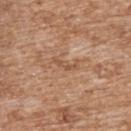{
  "biopsy_status": "not biopsied; imaged during a skin examination",
  "image": {
    "source": "total-body photography crop",
    "field_of_view_mm": 15
  },
  "lesion_size": {
    "long_diameter_mm_approx": 2.5
  },
  "lighting": "white-light",
  "patient": {
    "sex": "female",
    "age_approx": 70
  },
  "site": "upper back",
  "automated_metrics": {
    "eccentricity": 0.95,
    "shape_asymmetry": 0.55,
    "cielab_L": 53,
    "cielab_a": 20,
    "cielab_b": 33,
    "vs_skin_darker_L": 8.0,
    "vs_skin_contrast_norm": 5.5,
    "border_irregularity_0_10": 6.0,
    "color_variation_0_10": 0.0,
    "peripheral_color_asymmetry": 0.0
  }
}